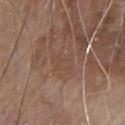| key | value |
|---|---|
| biopsy status | catalogued during a skin exam; not biopsied |
| imaging modality | ~15 mm tile from a whole-body skin photo |
| patient | male, aged approximately 80 |
| anatomic site | the chest |